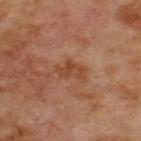Assessment:
This lesion was catalogued during total-body skin photography and was not selected for biopsy.
Context:
A 15 mm crop from a total-body photograph taken for skin-cancer surveillance. Captured under cross-polarized illumination. On the back. A male patient aged 68 to 72.